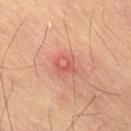<record>
<biopsy_status>not biopsied; imaged during a skin examination</biopsy_status>
<lighting>cross-polarized</lighting>
<image>
  <source>total-body photography crop</source>
  <field_of_view_mm>15</field_of_view_mm>
</image>
<automated_metrics>
  <border_irregularity_0_10>2.5</border_irregularity_0_10>
  <color_variation_0_10>7.0</color_variation_0_10>
  <peripheral_color_asymmetry>2.5</peripheral_color_asymmetry>
  <nevus_likeness_0_100>0</nevus_likeness_0_100>
</automated_metrics>
<patient>
  <sex>male</sex>
  <age_approx>65</age_approx>
</patient>
<site>lower back</site>
</record>